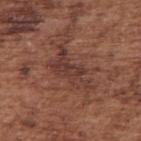Assessment:
No biopsy was performed on this lesion — it was imaged during a full skin examination and was not determined to be concerning.
Acquisition and patient details:
The lesion is on the right upper arm. A 15 mm close-up tile from a total-body photography series done for melanoma screening. The subject is a male aged 73 to 77. Imaged with white-light lighting. About 6 mm across.